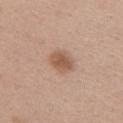{
  "biopsy_status": "not biopsied; imaged during a skin examination",
  "site": "back",
  "automated_metrics": {
    "vs_skin_darker_L": 11.0,
    "vs_skin_contrast_norm": 7.5,
    "border_irregularity_0_10": 1.0,
    "peripheral_color_asymmetry": 0.5,
    "nevus_likeness_0_100": 90,
    "lesion_detection_confidence_0_100": 100
  },
  "image": {
    "source": "total-body photography crop",
    "field_of_view_mm": 15
  },
  "lighting": "white-light",
  "patient": {
    "sex": "female",
    "age_approx": 40
  },
  "lesion_size": {
    "long_diameter_mm_approx": 3.0
  }
}